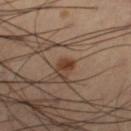Recorded during total-body skin imaging; not selected for excision or biopsy. Approximately 2 mm at its widest. Located on the left thigh. This image is a 15 mm lesion crop taken from a total-body photograph. Automated tile analysis of the lesion measured a shape eccentricity near 0.75 and a symmetry-axis asymmetry near 0.35. The analysis additionally found an automated nevus-likeness rating near 95 out of 100. Imaged with cross-polarized lighting. The subject is a male aged approximately 65.